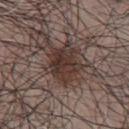Imaged during a routine full-body skin examination; the lesion was not biopsied and no histopathology is available.
A roughly 15 mm field-of-view crop from a total-body skin photograph.
On the front of the torso.
The lesion's longest dimension is about 4 mm.
The subject is a male aged 48–52.
The lesion-visualizer software estimated an automated nevus-likeness rating near 70 out of 100 and lesion-presence confidence of about 100/100.